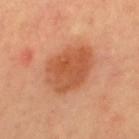| field | value |
|---|---|
| follow-up | total-body-photography surveillance lesion; no biopsy |
| location | the upper back |
| patient | male, approximately 55 years of age |
| image | 15 mm crop, total-body photography |